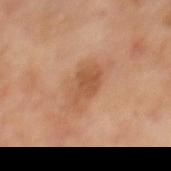notes = no biopsy performed (imaged during a skin exam); image source = total-body-photography crop, ~15 mm field of view; body site = the mid back; tile lighting = cross-polarized illumination; lesion diameter = ≈4.5 mm; subject = male, in their 70s.